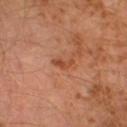<case>
<biopsy_status>not biopsied; imaged during a skin examination</biopsy_status>
<image>
  <source>total-body photography crop</source>
  <field_of_view_mm>15</field_of_view_mm>
</image>
<lesion_size>
  <long_diameter_mm_approx>2.5</long_diameter_mm_approx>
</lesion_size>
<site>left lower leg</site>
<lighting>cross-polarized</lighting>
<patient>
  <sex>male</sex>
  <age_approx>30</age_approx>
</patient>
</case>